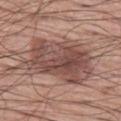Q: Was this lesion biopsied?
A: catalogued during a skin exam; not biopsied
Q: What is the anatomic site?
A: the left thigh
Q: What did automated image analysis measure?
A: a detector confidence of about 100 out of 100 that the crop contains a lesion
Q: What is the imaging modality?
A: total-body-photography crop, ~15 mm field of view
Q: Illumination type?
A: white-light illumination
Q: Lesion size?
A: ~8.5 mm (longest diameter)
Q: Patient demographics?
A: male, roughly 60 years of age